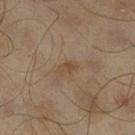A male subject aged approximately 65.
Approximately 2.5 mm at its widest.
A roughly 15 mm field-of-view crop from a total-body skin photograph.
From the right lower leg.
Automated image analysis of the tile measured a footprint of about 2.5 mm², a shape eccentricity near 0.85, and a symmetry-axis asymmetry near 0.4. The software also gave a border-irregularity rating of about 3.5/10, a within-lesion color-variation index near 1.5/10, and radial color variation of about 0.5.